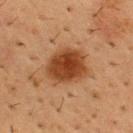<lesion>
  <patient>
    <sex>male</sex>
    <age_approx>55</age_approx>
  </patient>
  <image>
    <source>total-body photography crop</source>
    <field_of_view_mm>15</field_of_view_mm>
  </image>
  <site>chest</site>
  <lighting>cross-polarized</lighting>
</lesion>